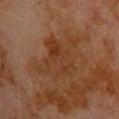Impression: The lesion was tiled from a total-body skin photograph and was not biopsied. Image and clinical context: A male subject, about 80 years old. Cropped from a total-body skin-imaging series; the visible field is about 15 mm. Located on the upper back.